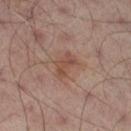Imaged during a routine full-body skin examination; the lesion was not biopsied and no histopathology is available. This is a cross-polarized tile. Automated image analysis of the tile measured about 7 CIELAB-L* units darker than the surrounding skin and a lesion-to-skin contrast of about 6.5 (normalized; higher = more distinct). It also reported a classifier nevus-likeness of about 0/100 and a lesion-detection confidence of about 100/100. The subject is a male in their mid- to late 60s. Located on the right thigh. About 3.5 mm across. A 15 mm close-up extracted from a 3D total-body photography capture.